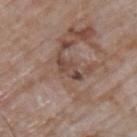  biopsy_status: not biopsied; imaged during a skin examination
  site: upper back
  patient:
    sex: male
    age_approx: 65
  image:
    source: total-body photography crop
    field_of_view_mm: 15
  lesion_size:
    long_diameter_mm_approx: 3.5